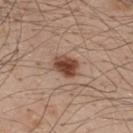Impression: Captured during whole-body skin photography for melanoma surveillance; the lesion was not biopsied. Acquisition and patient details: A male patient, approximately 50 years of age. Captured under white-light illumination. A lesion tile, about 15 mm wide, cut from a 3D total-body photograph. Located on the upper back.